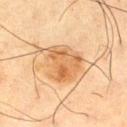Notes:
– notes: total-body-photography surveillance lesion; no biopsy
– patient: male, aged approximately 65
– imaging modality: total-body-photography crop, ~15 mm field of view
– anatomic site: the right thigh